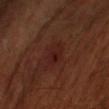Clinical impression: This lesion was catalogued during total-body skin photography and was not selected for biopsy. Image and clinical context: A lesion tile, about 15 mm wide, cut from a 3D total-body photograph. Located on the right upper arm. The lesion's longest dimension is about 3 mm. A male subject, approximately 70 years of age.